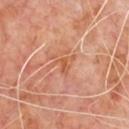Notes:
* follow-up · catalogued during a skin exam; not biopsied
* imaging modality · ~15 mm tile from a whole-body skin photo
* tile lighting · cross-polarized illumination
* site · the chest
* subject · male, aged 63 to 67
* size · ~3 mm (longest diameter)